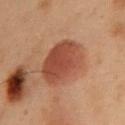follow-up = no biopsy performed (imaged during a skin exam)
subject = male, aged 53 to 57
acquisition = ~15 mm crop, total-body skin-cancer survey
lesion diameter = about 5.5 mm
body site = the chest
image-analysis metrics = an area of roughly 21 mm²; a normalized border contrast of about 8.5; a nevus-likeness score of about 100/100
tile lighting = cross-polarized